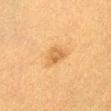Impression: This lesion was catalogued during total-body skin photography and was not selected for biopsy. Clinical summary: On the abdomen. This image is a 15 mm lesion crop taken from a total-body photograph. The lesion-visualizer software estimated a lesion area of about 5 mm². The software also gave an average lesion color of about L≈52 a*≈18 b*≈38 (CIELAB), about 8 CIELAB-L* units darker than the surrounding skin, and a lesion-to-skin contrast of about 6 (normalized; higher = more distinct). The software also gave an automated nevus-likeness rating near 20 out of 100 and a detector confidence of about 100 out of 100 that the crop contains a lesion. A female patient, aged approximately 40.